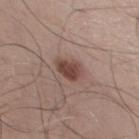Captured during whole-body skin photography for melanoma surveillance; the lesion was not biopsied.
The lesion's longest dimension is about 3 mm.
This image is a 15 mm lesion crop taken from a total-body photograph.
The lesion is on the upper back.
This is a white-light tile.
The patient is a male roughly 45 years of age.